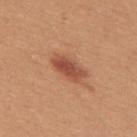No biopsy was performed on this lesion — it was imaged during a full skin examination and was not determined to be concerning. A 15 mm close-up tile from a total-body photography series done for melanoma screening. The recorded lesion diameter is about 4 mm. The total-body-photography lesion software estimated a mean CIELAB color near L≈50 a*≈27 b*≈32. A female patient, aged approximately 30. Located on the upper back.